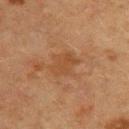Recorded during total-body skin imaging; not selected for excision or biopsy. The lesion is located on the upper back. The total-body-photography lesion software estimated a footprint of about 6.5 mm² and a symmetry-axis asymmetry near 0.25. And it measured a mean CIELAB color near L≈38 a*≈18 b*≈30, about 5 CIELAB-L* units darker than the surrounding skin, and a normalized lesion–skin contrast near 5.5. The lesion's longest dimension is about 3.5 mm. A female patient, aged around 55. A lesion tile, about 15 mm wide, cut from a 3D total-body photograph. This is a cross-polarized tile.